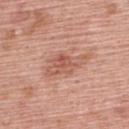<case>
  <biopsy_status>not biopsied; imaged during a skin examination</biopsy_status>
  <patient>
    <sex>male</sex>
    <age_approx>60</age_approx>
  </patient>
  <site>upper back</site>
  <lesion_size>
    <long_diameter_mm_approx>6.0</long_diameter_mm_approx>
  </lesion_size>
  <automated_metrics>
    <cielab_L>58</cielab_L>
    <cielab_a>24</cielab_a>
    <cielab_b>29</cielab_b>
    <vs_skin_darker_L>9.0</vs_skin_darker_L>
    <vs_skin_contrast_norm>6.0</vs_skin_contrast_norm>
  </automated_metrics>
  <lighting>white-light</lighting>
  <image>
    <source>total-body photography crop</source>
    <field_of_view_mm>15</field_of_view_mm>
  </image>
</case>